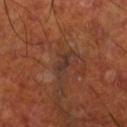{"biopsy_status": "not biopsied; imaged during a skin examination", "lighting": "cross-polarized", "patient": {"sex": "male", "age_approx": 70}, "image": {"source": "total-body photography crop", "field_of_view_mm": 15}, "site": "right lower leg"}